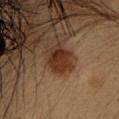Notes:
• workup: imaged on a skin check; not biopsied
• diameter: ≈2.5 mm
• lighting: cross-polarized
• location: the head or neck
• patient: female, aged 43 to 47
• image-analysis metrics: an area of roughly 5.5 mm², an eccentricity of roughly 0.5, and two-axis asymmetry of about 0.2; a lesion-to-skin contrast of about 10.5 (normalized; higher = more distinct); a nevus-likeness score of about 100/100 and a detector confidence of about 100 out of 100 that the crop contains a lesion
• acquisition: ~15 mm crop, total-body skin-cancer survey